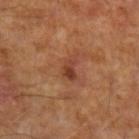This lesion was catalogued during total-body skin photography and was not selected for biopsy.
The lesion is on the left upper arm.
Longest diameter approximately 2.5 mm.
A lesion tile, about 15 mm wide, cut from a 3D total-body photograph.
A male patient, approximately 65 years of age.
Imaged with cross-polarized lighting.